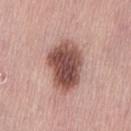No biopsy was performed on this lesion — it was imaged during a full skin examination and was not determined to be concerning.
A 15 mm close-up tile from a total-body photography series done for melanoma screening.
The lesion is located on the back.
The patient is a female about 65 years old.